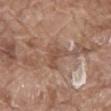Assessment: The lesion was tiled from a total-body skin photograph and was not biopsied. Context: On the mid back. Measured at roughly 3.5 mm in maximum diameter. A male patient aged 78–82. This image is a 15 mm lesion crop taken from a total-body photograph. Automated image analysis of the tile measured a normalized lesion–skin contrast near 6. And it measured a peripheral color-asymmetry measure near 1. The software also gave an automated nevus-likeness rating near 0 out of 100.